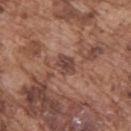Imaged during a routine full-body skin examination; the lesion was not biopsied and no histopathology is available.
The lesion is located on the upper back.
A close-up tile cropped from a whole-body skin photograph, about 15 mm across.
The subject is a male about 75 years old.
Captured under white-light illumination.
Automated tile analysis of the lesion measured a lesion area of about 4.5 mm² and an outline eccentricity of about 0.6 (0 = round, 1 = elongated). And it measured an average lesion color of about L≈43 a*≈21 b*≈24 (CIELAB), roughly 10 lightness units darker than nearby skin, and a normalized border contrast of about 8.